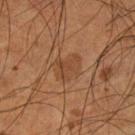Imaged during a routine full-body skin examination; the lesion was not biopsied and no histopathology is available. The lesion is located on the leg. A male patient approximately 55 years of age. A close-up tile cropped from a whole-body skin photograph, about 15 mm across.